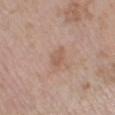biopsy status: imaged on a skin check; not biopsied
image source: ~15 mm crop, total-body skin-cancer survey
site: the left lower leg
subject: female, in their 70s